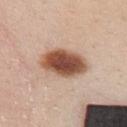Q: How was the tile lit?
A: white-light
Q: How was this image acquired?
A: total-body-photography crop, ~15 mm field of view
Q: Lesion location?
A: the mid back
Q: Patient demographics?
A: male, aged 23 to 27
Q: What is the histopathologic diagnosis?
A: a dysplastic (Clark) nevus